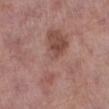| field | value |
|---|---|
| notes | total-body-photography surveillance lesion; no biopsy |
| lesion size | ~9.5 mm (longest diameter) |
| tile lighting | white-light illumination |
| image | 15 mm crop, total-body photography |
| patient | female, roughly 75 years of age |
| body site | the leg |